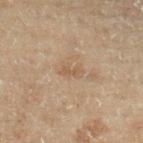workup=imaged on a skin check; not biopsied | site=the left lower leg | patient=male, aged around 70 | imaging modality=total-body-photography crop, ~15 mm field of view | image-analysis metrics=a footprint of about 3 mm² and two-axis asymmetry of about 0.35; a lesion color around L≈42 a*≈12 b*≈25 in CIELAB, about 5 CIELAB-L* units darker than the surrounding skin, and a normalized border contrast of about 5; a detector confidence of about 100 out of 100 that the crop contains a lesion.